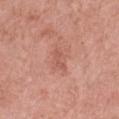The lesion was photographed on a routine skin check and not biopsied; there is no pathology result. A roughly 15 mm field-of-view crop from a total-body skin photograph. Measured at roughly 2.5 mm in maximum diameter. A female patient, aged 68–72. From the left thigh.